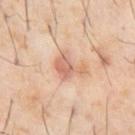workup = imaged on a skin check; not biopsied
lighting = cross-polarized
automated lesion analysis = a shape eccentricity near 0.7
site = the front of the torso
subject = male, aged around 60
imaging modality = ~15 mm crop, total-body skin-cancer survey